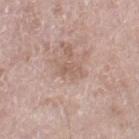No biopsy was performed on this lesion — it was imaged during a full skin examination and was not determined to be concerning. The subject is a female aged around 75. The lesion is located on the right thigh. Longest diameter approximately 2.5 mm. A 15 mm close-up tile from a total-body photography series done for melanoma screening.